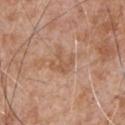Imaged during a routine full-body skin examination; the lesion was not biopsied and no histopathology is available.
Automated tile analysis of the lesion measured an area of roughly 6.5 mm², a shape eccentricity near 0.35, and two-axis asymmetry of about 0.4. The software also gave a classifier nevus-likeness of about 0/100 and a detector confidence of about 100 out of 100 that the crop contains a lesion.
A region of skin cropped from a whole-body photographic capture, roughly 15 mm wide.
A male patient in their mid-60s.
This is a white-light tile.
On the chest.